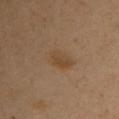The subject is a female roughly 40 years of age.
The lesion-visualizer software estimated a footprint of about 7.5 mm², an outline eccentricity of about 0.85 (0 = round, 1 = elongated), and a shape-asymmetry score of about 0.2 (0 = symmetric). The software also gave a lesion–skin lightness drop of about 6 and a normalized border contrast of about 6. And it measured a color-variation rating of about 3/10 and peripheral color asymmetry of about 1.
The lesion is located on the left upper arm.
Captured under cross-polarized illumination.
Cropped from a total-body skin-imaging series; the visible field is about 15 mm.
Measured at roughly 4.5 mm in maximum diameter.